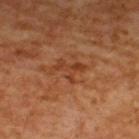Part of a total-body skin-imaging series; this lesion was reviewed on a skin check and was not flagged for biopsy.
A 15 mm close-up extracted from a 3D total-body photography capture.
From the upper back.
This is a cross-polarized tile.
Approximately 4 mm at its widest.
A male patient, in their 60s.